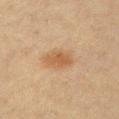Clinical impression:
Recorded during total-body skin imaging; not selected for excision or biopsy.
Context:
From the chest. An algorithmic analysis of the crop reported a mean CIELAB color near L≈50 a*≈17 b*≈33, a lesion–skin lightness drop of about 8, and a normalized lesion–skin contrast near 6.5. The software also gave a border-irregularity rating of about 2/10, internal color variation of about 3 on a 0–10 scale, and peripheral color asymmetry of about 1. Captured under cross-polarized illumination. Cropped from a total-body skin-imaging series; the visible field is about 15 mm. The patient is a female in their 40s. The recorded lesion diameter is about 4 mm.